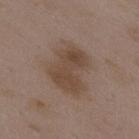<case>
<biopsy_status>not biopsied; imaged during a skin examination</biopsy_status>
<site>upper back</site>
<lesion_size>
  <long_diameter_mm_approx>5.5</long_diameter_mm_approx>
</lesion_size>
<patient>
  <sex>female</sex>
  <age_approx>35</age_approx>
</patient>
<lighting>white-light</lighting>
<automated_metrics>
  <cielab_L>44</cielab_L>
  <cielab_a>15</cielab_a>
  <cielab_b>26</cielab_b>
  <vs_skin_contrast_norm>7.5</vs_skin_contrast_norm>
  <nevus_likeness_0_100>5</nevus_likeness_0_100>
</automated_metrics>
<image>
  <source>total-body photography crop</source>
  <field_of_view_mm>15</field_of_view_mm>
</image>
</case>